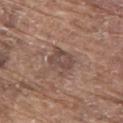follow-up: no biopsy performed (imaged during a skin exam) | patient: male, roughly 80 years of age | body site: the upper back | image: ~15 mm crop, total-body skin-cancer survey | automated lesion analysis: a mean CIELAB color near L≈47 a*≈17 b*≈22, about 8 CIELAB-L* units darker than the surrounding skin, and a lesion-to-skin contrast of about 6.5 (normalized; higher = more distinct).